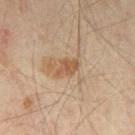The lesion was photographed on a routine skin check and not biopsied; there is no pathology result. A lesion tile, about 15 mm wide, cut from a 3D total-body photograph. Automated tile analysis of the lesion measured an area of roughly 4 mm², an outline eccentricity of about 0.75 (0 = round, 1 = elongated), and two-axis asymmetry of about 0.3. The software also gave a border-irregularity index near 3/10, a within-lesion color-variation index near 1.5/10, and a peripheral color-asymmetry measure near 0.5. From the leg. A subject aged 53–57. The tile uses cross-polarized illumination.